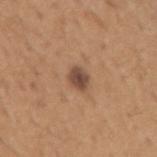{"biopsy_status": "not biopsied; imaged during a skin examination", "lesion_size": {"long_diameter_mm_approx": 2.5}, "image": {"source": "total-body photography crop", "field_of_view_mm": 15}, "lighting": "white-light", "automated_metrics": {"cielab_L": 47, "cielab_a": 19, "cielab_b": 28, "vs_skin_contrast_norm": 9.5, "border_irregularity_0_10": 1.5, "color_variation_0_10": 2.5, "peripheral_color_asymmetry": 0.5, "nevus_likeness_0_100": 65, "lesion_detection_confidence_0_100": 100}, "site": "arm", "patient": {"sex": "male", "age_approx": 65}}